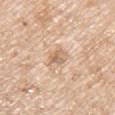Imaged during a routine full-body skin examination; the lesion was not biopsied and no histopathology is available. A roughly 15 mm field-of-view crop from a total-body skin photograph. Automated image analysis of the tile measured a border-irregularity index near 2.5/10, a within-lesion color-variation index near 4.5/10, and a peripheral color-asymmetry measure near 1.5. The software also gave a nevus-likeness score of about 0/100 and a lesion-detection confidence of about 100/100. A male subject aged 63 to 67. About 2.5 mm across. The tile uses white-light illumination. On the left upper arm.